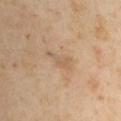– acquisition · ~15 mm crop, total-body skin-cancer survey
– location · the arm
– patient · male, in their 40s